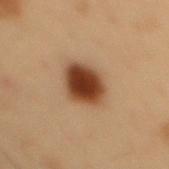This is a cross-polarized tile. Located on the mid back. A 15 mm close-up extracted from a 3D total-body photography capture. A male patient in their mid- to late 50s.